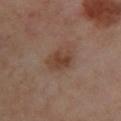Q: Was a biopsy performed?
A: catalogued during a skin exam; not biopsied
Q: What is the lesion's diameter?
A: ≈3.5 mm
Q: Patient demographics?
A: female, roughly 55 years of age
Q: Illumination type?
A: cross-polarized illumination
Q: Automated lesion metrics?
A: an outline eccentricity of about 0.7 (0 = round, 1 = elongated) and a shape-asymmetry score of about 0.2 (0 = symmetric); an average lesion color of about L≈42 a*≈18 b*≈27 (CIELAB) and a normalized lesion–skin contrast near 7.5; a border-irregularity rating of about 2.5/10 and a peripheral color-asymmetry measure near 1
Q: How was this image acquired?
A: ~15 mm crop, total-body skin-cancer survey
Q: Where on the body is the lesion?
A: the left lower leg